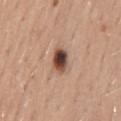* follow-up: imaged on a skin check; not biopsied
* image-analysis metrics: a color-variation rating of about 8.5/10 and peripheral color asymmetry of about 2.5; lesion-presence confidence of about 100/100
* lesion diameter: ≈3 mm
* body site: the abdomen
* subject: female, aged 33–37
* acquisition: ~15 mm crop, total-body skin-cancer survey